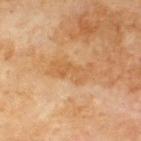Q: Was a biopsy performed?
A: no biopsy performed (imaged during a skin exam)
Q: Illumination type?
A: cross-polarized
Q: Lesion location?
A: the upper back
Q: Who is the patient?
A: male, approximately 70 years of age
Q: How was this image acquired?
A: 15 mm crop, total-body photography
Q: How large is the lesion?
A: ~5 mm (longest diameter)